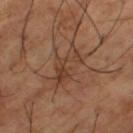{
  "biopsy_status": "not biopsied; imaged during a skin examination",
  "lesion_size": {
    "long_diameter_mm_approx": 5.0
  },
  "image": {
    "source": "total-body photography crop",
    "field_of_view_mm": 15
  },
  "site": "left thigh",
  "patient": {
    "sex": "male",
    "age_approx": 65
  }
}